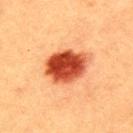Recorded during total-body skin imaging; not selected for excision or biopsy. Captured under cross-polarized illumination. The lesion's longest dimension is about 6 mm. The lesion is on the upper back. An algorithmic analysis of the crop reported a lesion area of about 17 mm² and a shape eccentricity near 0.7. It also reported a lesion color around L≈43 a*≈32 b*≈36 in CIELAB and a normalized lesion–skin contrast near 13. And it measured border irregularity of about 1.5 on a 0–10 scale, a color-variation rating of about 7/10, and radial color variation of about 2.5. It also reported a classifier nevus-likeness of about 100/100 and a lesion-detection confidence of about 100/100. A 15 mm close-up extracted from a 3D total-body photography capture. A male subject, about 40 years old.